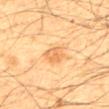Part of a total-body skin-imaging series; this lesion was reviewed on a skin check and was not flagged for biopsy.
A region of skin cropped from a whole-body photographic capture, roughly 15 mm wide.
A male subject approximately 65 years of age.
From the mid back.
The total-body-photography lesion software estimated an average lesion color of about L≈58 a*≈20 b*≈39 (CIELAB) and about 8 CIELAB-L* units darker than the surrounding skin. And it measured a border-irregularity index near 2/10, a within-lesion color-variation index near 2/10, and peripheral color asymmetry of about 0.5.
Longest diameter approximately 3 mm.
This is a cross-polarized tile.